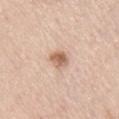The lesion was photographed on a routine skin check and not biopsied; there is no pathology result. Cropped from a whole-body photographic skin survey; the tile spans about 15 mm. The patient is a male aged around 65. From the right thigh.